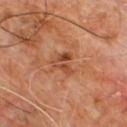biopsy status: catalogued during a skin exam; not biopsied
acquisition: total-body-photography crop, ~15 mm field of view
tile lighting: cross-polarized illumination
size: ≈3 mm
patient: male, in their 60s
automated lesion analysis: a footprint of about 5 mm² and a symmetry-axis asymmetry near 0.5; a lesion–skin lightness drop of about 9 and a lesion-to-skin contrast of about 7 (normalized; higher = more distinct)
site: the upper back